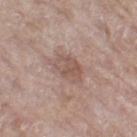Notes:
- biopsy status · total-body-photography surveillance lesion; no biopsy
- site · the leg
- size · ≈3.5 mm
- imaging modality · 15 mm crop, total-body photography
- patient · female, aged approximately 75